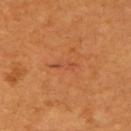Captured during whole-body skin photography for melanoma surveillance; the lesion was not biopsied. The total-body-photography lesion software estimated an average lesion color of about L≈50 a*≈29 b*≈39 (CIELAB). The software also gave a border-irregularity rating of about 5.5/10, a color-variation rating of about 0/10, and a peripheral color-asymmetry measure near 0. This is a cross-polarized tile. A 15 mm close-up extracted from a 3D total-body photography capture. A male subject about 65 years old. Located on the left upper arm.